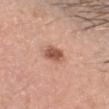Assessment:
The lesion was tiled from a total-body skin photograph and was not biopsied.
Clinical summary:
Cropped from a whole-body photographic skin survey; the tile spans about 15 mm. A male subject aged 33–37. The lesion is on the head or neck.